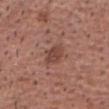Assessment:
Part of a total-body skin-imaging series; this lesion was reviewed on a skin check and was not flagged for biopsy.
Context:
The patient is a male aged approximately 65. A close-up tile cropped from a whole-body skin photograph, about 15 mm across. The lesion is on the front of the torso.